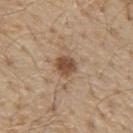  biopsy_status: not biopsied; imaged during a skin examination
  site: chest
  lesion_size:
    long_diameter_mm_approx: 2.5
  image:
    source: total-body photography crop
    field_of_view_mm: 15
  patient:
    sex: male
    age_approx: 70
  automated_metrics:
    cielab_L: 48
    cielab_a: 18
    cielab_b: 30
    vs_skin_contrast_norm: 9.5
  lighting: white-light